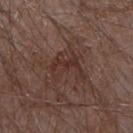subject = male, in their mid- to late 70s
image-analysis metrics = a lesion area of about 9.5 mm², an eccentricity of roughly 0.75, and a symmetry-axis asymmetry near 0.6; a mean CIELAB color near L≈33 a*≈18 b*≈22, a lesion–skin lightness drop of about 6, and a normalized border contrast of about 6; a border-irregularity index near 7.5/10, a color-variation rating of about 3.5/10, and peripheral color asymmetry of about 1.5
site = the arm
image = ~15 mm tile from a whole-body skin photo